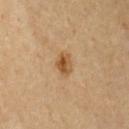Case summary:
- follow-up: catalogued during a skin exam; not biopsied
- lighting: cross-polarized illumination
- site: the front of the torso
- imaging modality: 15 mm crop, total-body photography
- patient: male, aged 58–62
- image-analysis metrics: an area of roughly 4.5 mm², an outline eccentricity of about 0.7 (0 = round, 1 = elongated), and two-axis asymmetry of about 0.2; border irregularity of about 2 on a 0–10 scale, a within-lesion color-variation index near 5/10, and radial color variation of about 2
- lesion size: about 2.5 mm